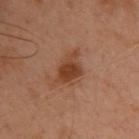{"biopsy_status": "not biopsied; imaged during a skin examination", "site": "upper back", "patient": {"sex": "female", "age_approx": 55}, "image": {"source": "total-body photography crop", "field_of_view_mm": 15}}